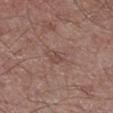Part of a total-body skin-imaging series; this lesion was reviewed on a skin check and was not flagged for biopsy. A male patient roughly 55 years of age. The lesion is located on the right lower leg. A region of skin cropped from a whole-body photographic capture, roughly 15 mm wide. Imaged with white-light lighting. Approximately 3 mm at its widest.